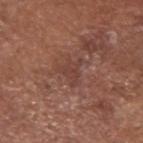Impression: Part of a total-body skin-imaging series; this lesion was reviewed on a skin check and was not flagged for biopsy. Context: On the head or neck. Approximately 3 mm at its widest. Automated tile analysis of the lesion measured a classifier nevus-likeness of about 0/100. A male patient, aged 78 to 82. Imaged with white-light lighting. A lesion tile, about 15 mm wide, cut from a 3D total-body photograph.